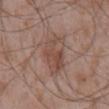Case summary:
* notes: total-body-photography surveillance lesion; no biopsy
* patient: male, about 50 years old
* imaging modality: 15 mm crop, total-body photography
* diameter: ≈6 mm
* tile lighting: white-light
* location: the mid back
* TBP lesion metrics: an area of roughly 13 mm², a shape eccentricity near 0.9, and two-axis asymmetry of about 0.2; internal color variation of about 5.5 on a 0–10 scale and a peripheral color-asymmetry measure near 2; a classifier nevus-likeness of about 0/100 and a detector confidence of about 100 out of 100 that the crop contains a lesion